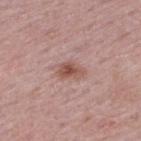workup: total-body-photography surveillance lesion; no biopsy | automated lesion analysis: two-axis asymmetry of about 0.3; an average lesion color of about L≈52 a*≈22 b*≈24 (CIELAB), a lesion–skin lightness drop of about 11, and a normalized border contrast of about 8; border irregularity of about 3 on a 0–10 scale, a color-variation rating of about 4/10, and radial color variation of about 1; a nevus-likeness score of about 70/100 and lesion-presence confidence of about 100/100 | subject: female, about 50 years old | diameter: ~3.5 mm (longest diameter) | location: the upper back | image source: total-body-photography crop, ~15 mm field of view.